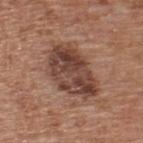Assessment: The lesion was photographed on a routine skin check and not biopsied; there is no pathology result. Acquisition and patient details: The subject is a male aged 63–67. The lesion is on the upper back. A 15 mm close-up tile from a total-body photography series done for melanoma screening.